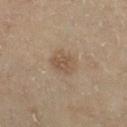Part of a total-body skin-imaging series; this lesion was reviewed on a skin check and was not flagged for biopsy.